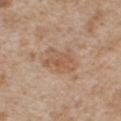Case summary:
* notes — imaged on a skin check; not biopsied
* site — the chest
* diameter — ≈4 mm
* patient — male, roughly 65 years of age
* imaging modality — ~15 mm crop, total-body skin-cancer survey